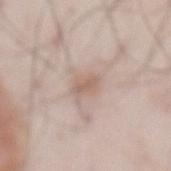image:
  source: total-body photography crop
  field_of_view_mm: 15
lesion_size:
  long_diameter_mm_approx: 2.5
patient:
  sex: male
  age_approx: 55
site: front of the torso